Cropped from a total-body skin-imaging series; the visible field is about 15 mm. The subject is a female in their mid- to late 60s. On the right lower leg:
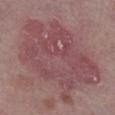<lesion>
<lighting>white-light</lighting>
<automated_metrics>
  <area_mm2_approx>65.0</area_mm2_approx>
  <eccentricity>0.8</eccentricity>
  <shape_asymmetry>0.3</shape_asymmetry>
  <cielab_L>47</cielab_L>
  <cielab_a>23</cielab_a>
  <cielab_b>17</cielab_b>
  <vs_skin_darker_L>8.0</vs_skin_darker_L>
  <vs_skin_contrast_norm>6.0</vs_skin_contrast_norm>
  <nevus_likeness_0_100>0</nevus_likeness_0_100>
</automated_metrics>
<lesion_size>
  <long_diameter_mm_approx>12.5</long_diameter_mm_approx>
</lesion_size>
<diagnosis>
  <histopathology>lichen planus-like keratosis</histopathology>
  <malignancy>benign</malignancy>
  <taxonomic_path>Benign, Benign epidermal proliferations, Lichen planus like keratosis</taxonomic_path>
</diagnosis>
</lesion>